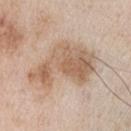follow-up = no biopsy performed (imaged during a skin exam) | location = the left upper arm | image source = ~15 mm tile from a whole-body skin photo | lesion diameter = ~8 mm (longest diameter) | lighting = white-light | patient = male, about 55 years old.